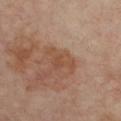Impression: The lesion was photographed on a routine skin check and not biopsied; there is no pathology result. Acquisition and patient details: Located on the front of the torso. The patient is roughly 55 years of age. A lesion tile, about 15 mm wide, cut from a 3D total-body photograph. Automated tile analysis of the lesion measured a lesion color around L≈47 a*≈20 b*≈29 in CIELAB and a lesion-to-skin contrast of about 6 (normalized; higher = more distinct). And it measured an automated nevus-likeness rating near 0 out of 100 and a lesion-detection confidence of about 100/100. The tile uses cross-polarized illumination.